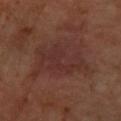Assessment: The lesion was photographed on a routine skin check and not biopsied; there is no pathology result. Context: The subject is a female roughly 50 years of age. The lesion is located on the right forearm. This is a cross-polarized tile. About 7.5 mm across. A 15 mm crop from a total-body photograph taken for skin-cancer surveillance.